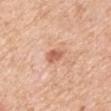Q: Was a biopsy performed?
A: total-body-photography surveillance lesion; no biopsy
Q: How was this image acquired?
A: 15 mm crop, total-body photography
Q: How large is the lesion?
A: ≈3 mm
Q: Who is the patient?
A: male, roughly 50 years of age
Q: Where on the body is the lesion?
A: the right upper arm
Q: What lighting was used for the tile?
A: white-light illumination